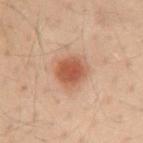lesion diameter: ~3.5 mm (longest diameter) | illumination: cross-polarized | site: the back | image: 15 mm crop, total-body photography | patient: male, about 40 years old.